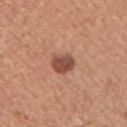Findings:
- follow-up — no biopsy performed (imaged during a skin exam)
- anatomic site — the left upper arm
- lighting — white-light illumination
- lesion diameter — about 3 mm
- acquisition — total-body-photography crop, ~15 mm field of view
- TBP lesion metrics — an area of roughly 6 mm² and two-axis asymmetry of about 0.15; a border-irregularity index near 1.5/10 and radial color variation of about 1.5; a nevus-likeness score of about 85/100 and a detector confidence of about 100 out of 100 that the crop contains a lesion
- subject — female, aged 33–37